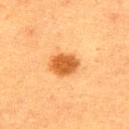Part of a total-body skin-imaging series; this lesion was reviewed on a skin check and was not flagged for biopsy.
Automated tile analysis of the lesion measured a footprint of about 8.5 mm², an outline eccentricity of about 0.7 (0 = round, 1 = elongated), and two-axis asymmetry of about 0.2. The software also gave border irregularity of about 2 on a 0–10 scale, a within-lesion color-variation index near 3/10, and peripheral color asymmetry of about 1.
About 4 mm across.
A 15 mm close-up tile from a total-body photography series done for melanoma screening.
The lesion is on the upper back.
This is a cross-polarized tile.
A male patient aged around 40.